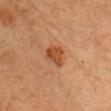biopsy status: imaged on a skin check; not biopsied
lighting: cross-polarized illumination
image source: total-body-photography crop, ~15 mm field of view
subject: female, aged 63 to 67
lesion size: about 3 mm
anatomic site: the head or neck